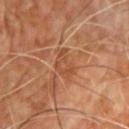| field | value |
|---|---|
| notes | no biopsy performed (imaged during a skin exam) |
| lesion size | ≈4 mm |
| imaging modality | total-body-photography crop, ~15 mm field of view |
| tile lighting | cross-polarized illumination |
| patient | male, aged 53 to 57 |
| anatomic site | the chest |